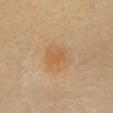No biopsy was performed on this lesion — it was imaged during a full skin examination and was not determined to be concerning.
Automated image analysis of the tile measured a footprint of about 5 mm², a shape eccentricity near 0.6, and two-axis asymmetry of about 0.2. And it measured a lesion color around L≈52 a*≈18 b*≈36 in CIELAB, roughly 6 lightness units darker than nearby skin, and a normalized border contrast of about 5.5. It also reported border irregularity of about 2.5 on a 0–10 scale and radial color variation of about 0.5. The software also gave an automated nevus-likeness rating near 85 out of 100.
Imaged with cross-polarized lighting.
The lesion is on the front of the torso.
Longest diameter approximately 2.5 mm.
A female subject approximately 60 years of age.
A lesion tile, about 15 mm wide, cut from a 3D total-body photograph.